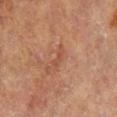Impression:
The lesion was photographed on a routine skin check and not biopsied; there is no pathology result.
Image and clinical context:
Automated tile analysis of the lesion measured roughly 7 lightness units darker than nearby skin and a normalized border contrast of about 5. The recorded lesion diameter is about 2.5 mm. The lesion is located on the left lower leg. A female patient aged 73–77. Imaged with cross-polarized lighting. A region of skin cropped from a whole-body photographic capture, roughly 15 mm wide.